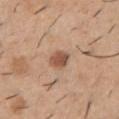Assessment: Part of a total-body skin-imaging series; this lesion was reviewed on a skin check and was not flagged for biopsy. Acquisition and patient details: Imaged with white-light lighting. The subject is a male about 40 years old. Cropped from a total-body skin-imaging series; the visible field is about 15 mm. On the front of the torso.